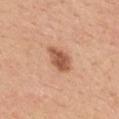No biopsy was performed on this lesion — it was imaged during a full skin examination and was not determined to be concerning.
Cropped from a total-body skin-imaging series; the visible field is about 15 mm.
From the upper back.
Imaged with white-light lighting.
The subject is a female aged approximately 45.